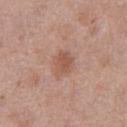{"biopsy_status": "not biopsied; imaged during a skin examination", "lighting": "white-light", "patient": {"sex": "female", "age_approx": 40}, "image": {"source": "total-body photography crop", "field_of_view_mm": 15}, "automated_metrics": {"border_irregularity_0_10": 2.0, "color_variation_0_10": 2.5, "peripheral_color_asymmetry": 1.0}, "site": "right thigh"}